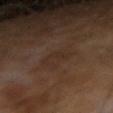Context:
Cropped from a total-body skin-imaging series; the visible field is about 15 mm. Captured under cross-polarized illumination. The subject is a male aged approximately 65. The total-body-photography lesion software estimated a footprint of about 4 mm² and a shape eccentricity near 0.8. The analysis additionally found a lesion color around L≈27 a*≈15 b*≈22 in CIELAB, roughly 4 lightness units darker than nearby skin, and a lesion-to-skin contrast of about 4.5 (normalized; higher = more distinct). And it measured a classifier nevus-likeness of about 0/100 and a detector confidence of about 100 out of 100 that the crop contains a lesion. Located on the right forearm. The lesion's longest dimension is about 3 mm.